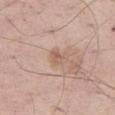Context:
Cropped from a total-body skin-imaging series; the visible field is about 15 mm. This is a white-light tile. An algorithmic analysis of the crop reported a border-irregularity index near 2.5/10 and a color-variation rating of about 1/10. A male subject, aged 53–57. On the right thigh.